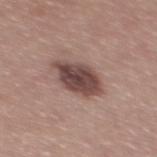Assessment:
The lesion was tiled from a total-body skin photograph and was not biopsied.
Context:
A close-up tile cropped from a whole-body skin photograph, about 15 mm across. An algorithmic analysis of the crop reported a lesion area of about 13 mm² and a shape-asymmetry score of about 0.15 (0 = symmetric). And it measured an automated nevus-likeness rating near 35 out of 100 and lesion-presence confidence of about 100/100. The patient is a male about 40 years old. The lesion is located on the mid back. The lesion's longest dimension is about 5 mm. Captured under white-light illumination.